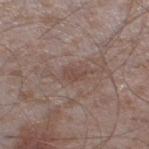Findings:
– notes · catalogued during a skin exam; not biopsied
– lesion diameter · about 2.5 mm
– automated lesion analysis · a footprint of about 3.5 mm², an outline eccentricity of about 0.8 (0 = round, 1 = elongated), and a shape-asymmetry score of about 0.2 (0 = symmetric); a nevus-likeness score of about 0/100 and a lesion-detection confidence of about 100/100
– image source · total-body-photography crop, ~15 mm field of view
– location · the left thigh
– subject · male, aged around 45